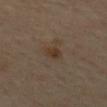image-analysis metrics: a footprint of about 3 mm², an outline eccentricity of about 0.8 (0 = round, 1 = elongated), and a shape-asymmetry score of about 0.2 (0 = symmetric); a border-irregularity rating of about 2/10, a color-variation rating of about 2.5/10, and a peripheral color-asymmetry measure near 1
imaging modality: 15 mm crop, total-body photography
body site: the back
patient: male, about 65 years old
lesion diameter: ~2.5 mm (longest diameter)